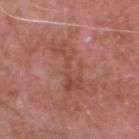Notes:
* follow-up: catalogued during a skin exam; not biopsied
* image source: total-body-photography crop, ~15 mm field of view
* lesion size: ≈6 mm
* patient: male, approximately 75 years of age
* body site: the head or neck
* image-analysis metrics: a lesion color around L≈48 a*≈24 b*≈28 in CIELAB, roughly 6 lightness units darker than nearby skin, and a normalized lesion–skin contrast near 5.5; a color-variation rating of about 2.5/10 and a peripheral color-asymmetry measure near 1